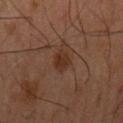This lesion was catalogued during total-body skin photography and was not selected for biopsy. The lesion is located on the mid back. This image is a 15 mm lesion crop taken from a total-body photograph. Captured under cross-polarized illumination. A male subject roughly 60 years of age. Longest diameter approximately 3 mm.